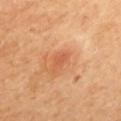body site: the mid back
image-analysis metrics: a footprint of about 3 mm², a shape eccentricity near 0.8, and a shape-asymmetry score of about 0.3 (0 = symmetric); a lesion color around L≈57 a*≈29 b*≈38 in CIELAB, about 7 CIELAB-L* units darker than the surrounding skin, and a lesion-to-skin contrast of about 4.5 (normalized; higher = more distinct); a nevus-likeness score of about 0/100 and lesion-presence confidence of about 100/100
lighting: cross-polarized illumination
patient: female, in their mid-60s
image: ~15 mm tile from a whole-body skin photo
diameter: ~2.5 mm (longest diameter)